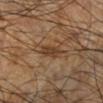<lesion>
<biopsy_status>not biopsied; imaged during a skin examination</biopsy_status>
<lighting>cross-polarized</lighting>
<patient>
  <sex>male</sex>
  <age_approx>60</age_approx>
</patient>
<site>left lower leg</site>
<image>
  <source>total-body photography crop</source>
  <field_of_view_mm>15</field_of_view_mm>
</image>
<lesion_size>
  <long_diameter_mm_approx>3.5</long_diameter_mm_approx>
</lesion_size>
<automated_metrics>
  <vs_skin_darker_L>9.0</vs_skin_darker_L>
  <border_irregularity_0_10>2.5</border_irregularity_0_10>
  <color_variation_0_10>1.5</color_variation_0_10>
  <nevus_likeness_0_100>50</nevus_likeness_0_100>
  <lesion_detection_confidence_0_100>95</lesion_detection_confidence_0_100>
</automated_metrics>
</lesion>